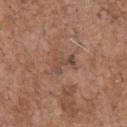Impression:
Part of a total-body skin-imaging series; this lesion was reviewed on a skin check and was not flagged for biopsy.
Image and clinical context:
The lesion is located on the chest. A 15 mm crop from a total-body photograph taken for skin-cancer surveillance. A male subject, in their 70s.